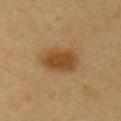No biopsy was performed on this lesion — it was imaged during a full skin examination and was not determined to be concerning.
A lesion tile, about 15 mm wide, cut from a 3D total-body photograph.
From the chest.
A male subject roughly 60 years of age.
The total-body-photography lesion software estimated a mean CIELAB color near L≈45 a*≈20 b*≈38.
Captured under cross-polarized illumination.
Approximately 4.5 mm at its widest.